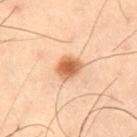The lesion was photographed on a routine skin check and not biopsied; there is no pathology result.